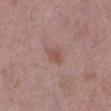biopsy_status: not biopsied; imaged during a skin examination
lesion_size:
  long_diameter_mm_approx: 3.0
automated_metrics:
  cielab_L: 52
  cielab_a: 20
  cielab_b: 24
  nevus_likeness_0_100: 5
  lesion_detection_confidence_0_100: 100
lighting: white-light
patient:
  sex: female
  age_approx: 65
image:
  source: total-body photography crop
  field_of_view_mm: 15
site: leg